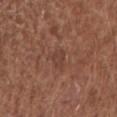Case summary:
– biopsy status · catalogued during a skin exam; not biopsied
– tile lighting · white-light illumination
– site · the right upper arm
– image-analysis metrics · an area of roughly 4 mm², an outline eccentricity of about 0.55 (0 = round, 1 = elongated), and a symmetry-axis asymmetry near 0.35; a mean CIELAB color near L≈40 a*≈21 b*≈26, about 6 CIELAB-L* units darker than the surrounding skin, and a lesion-to-skin contrast of about 5 (normalized; higher = more distinct); a nevus-likeness score of about 0/100
– lesion diameter · ~2.5 mm (longest diameter)
– image · total-body-photography crop, ~15 mm field of view
– subject · female, aged 48 to 52